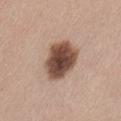Findings:
• notes: total-body-photography surveillance lesion; no biopsy
• TBP lesion metrics: an automated nevus-likeness rating near 80 out of 100
• patient: female, aged around 40
• illumination: white-light
• acquisition: total-body-photography crop, ~15 mm field of view
• site: the lower back